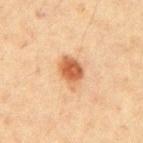– follow-up · no biopsy performed (imaged during a skin exam)
– site · the arm
– patient · female, aged around 40
– image · total-body-photography crop, ~15 mm field of view